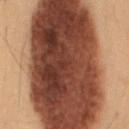patient:
  sex: male
  age_approx: 55
site: lower back
image:
  source: total-body photography crop
  field_of_view_mm: 15
lighting: cross-polarized
lesion_size:
  long_diameter_mm_approx: 19.5